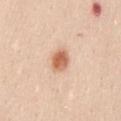This lesion was catalogued during total-body skin photography and was not selected for biopsy. The tile uses white-light illumination. About 3 mm across. The patient is a female aged approximately 30. Cropped from a whole-body photographic skin survey; the tile spans about 15 mm. The lesion-visualizer software estimated a footprint of about 5.5 mm², an outline eccentricity of about 0.5 (0 = round, 1 = elongated), and a symmetry-axis asymmetry near 0.15. The software also gave an average lesion color of about L≈64 a*≈24 b*≈35 (CIELAB), about 14 CIELAB-L* units darker than the surrounding skin, and a lesion-to-skin contrast of about 9 (normalized; higher = more distinct). It also reported a border-irregularity rating of about 1.5/10, internal color variation of about 4 on a 0–10 scale, and radial color variation of about 1. The software also gave an automated nevus-likeness rating near 100 out of 100 and a lesion-detection confidence of about 100/100. On the mid back.